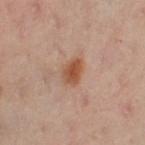Captured during whole-body skin photography for melanoma surveillance; the lesion was not biopsied.